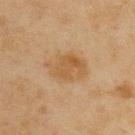Impression: This lesion was catalogued during total-body skin photography and was not selected for biopsy. Acquisition and patient details: The lesion is on the upper back. Captured under cross-polarized illumination. Longest diameter approximately 5 mm. A lesion tile, about 15 mm wide, cut from a 3D total-body photograph. A male patient aged 43 to 47.